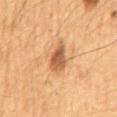No biopsy was performed on this lesion — it was imaged during a full skin examination and was not determined to be concerning. Longest diameter approximately 4.5 mm. The lesion is on the mid back. A male subject roughly 65 years of age. An algorithmic analysis of the crop reported an average lesion color of about L≈47 a*≈19 b*≈32 (CIELAB) and a lesion–skin lightness drop of about 12. It also reported border irregularity of about 3.5 on a 0–10 scale, a color-variation rating of about 4.5/10, and a peripheral color-asymmetry measure near 1.5. Cropped from a total-body skin-imaging series; the visible field is about 15 mm. Captured under cross-polarized illumination.